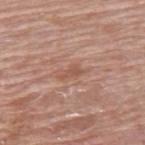Q: Was a biopsy performed?
A: no biopsy performed (imaged during a skin exam)
Q: How was this image acquired?
A: ~15 mm crop, total-body skin-cancer survey
Q: What is the lesion's diameter?
A: about 2.5 mm
Q: Who is the patient?
A: male, in their 80s
Q: Lesion location?
A: the upper back
Q: How was the tile lit?
A: white-light illumination